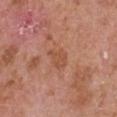  biopsy_status: not biopsied; imaged during a skin examination
  image:
    source: total-body photography crop
    field_of_view_mm: 15
  lighting: white-light
  site: arm
  patient:
    sex: male
    age_approx: 65